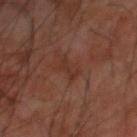| field | value |
|---|---|
| follow-up | no biopsy performed (imaged during a skin exam) |
| anatomic site | the upper back |
| illumination | cross-polarized |
| size | about 3.5 mm |
| image | total-body-photography crop, ~15 mm field of view |
| subject | male, roughly 60 years of age |